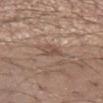| key | value |
|---|---|
| follow-up | catalogued during a skin exam; not biopsied |
| subject | male, aged 28–32 |
| image-analysis metrics | a footprint of about 3 mm², an eccentricity of roughly 0.85, and two-axis asymmetry of about 0.4; a border-irregularity rating of about 4/10, internal color variation of about 1.5 on a 0–10 scale, and peripheral color asymmetry of about 0.5 |
| location | the left forearm |
| lighting | white-light |
| imaging modality | ~15 mm tile from a whole-body skin photo |
| lesion diameter | ≈3 mm |